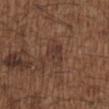Captured during whole-body skin photography for melanoma surveillance; the lesion was not biopsied.
Located on the chest.
A roughly 15 mm field-of-view crop from a total-body skin photograph.
The subject is a male approximately 50 years of age.